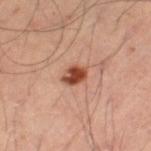  biopsy_status: not biopsied; imaged during a skin examination
  site: left thigh
  lighting: cross-polarized
  patient:
    sex: male
    age_approx: 50
  image:
    source: total-body photography crop
    field_of_view_mm: 15
  lesion_size:
    long_diameter_mm_approx: 3.0